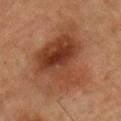Q: Was this lesion biopsied?
A: no biopsy performed (imaged during a skin exam)
Q: Lesion size?
A: about 9 mm
Q: How was the tile lit?
A: cross-polarized
Q: Lesion location?
A: the chest
Q: What are the patient's age and sex?
A: male, roughly 55 years of age
Q: What is the imaging modality?
A: total-body-photography crop, ~15 mm field of view
Q: Automated lesion metrics?
A: border irregularity of about 6 on a 0–10 scale